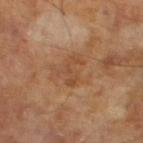Clinical impression:
This lesion was catalogued during total-body skin photography and was not selected for biopsy.
Acquisition and patient details:
The patient is a male aged 63 to 67. Measured at roughly 3.5 mm in maximum diameter. An algorithmic analysis of the crop reported a footprint of about 5 mm², an eccentricity of roughly 0.8, and a shape-asymmetry score of about 0.65 (0 = symmetric). It also reported a mean CIELAB color near L≈46 a*≈21 b*≈33, about 6 CIELAB-L* units darker than the surrounding skin, and a normalized border contrast of about 5. The software also gave a border-irregularity rating of about 9/10, internal color variation of about 0 on a 0–10 scale, and a peripheral color-asymmetry measure near 0. It also reported a detector confidence of about 100 out of 100 that the crop contains a lesion. The tile uses cross-polarized illumination. A 15 mm crop from a total-body photograph taken for skin-cancer surveillance.